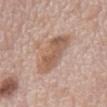Impression:
The lesion was tiled from a total-body skin photograph and was not biopsied.
Acquisition and patient details:
The lesion's longest dimension is about 7 mm. Captured under white-light illumination. A male patient roughly 70 years of age. A lesion tile, about 15 mm wide, cut from a 3D total-body photograph. The total-body-photography lesion software estimated a lesion area of about 16 mm² and a symmetry-axis asymmetry near 0.25. The analysis additionally found a classifier nevus-likeness of about 0/100 and a lesion-detection confidence of about 100/100. The lesion is on the mid back.